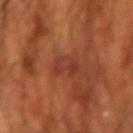workup: total-body-photography surveillance lesion; no biopsy | subject: male, roughly 70 years of age | lighting: cross-polarized illumination | body site: the left forearm | image: ~15 mm crop, total-body skin-cancer survey.